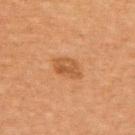Q: Was this lesion biopsied?
A: imaged on a skin check; not biopsied
Q: Where on the body is the lesion?
A: the back
Q: Lesion size?
A: ~3.5 mm (longest diameter)
Q: Who is the patient?
A: female, aged around 60
Q: Illumination type?
A: cross-polarized illumination
Q: What kind of image is this?
A: 15 mm crop, total-body photography